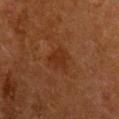This lesion was catalogued during total-body skin photography and was not selected for biopsy.
The tile uses cross-polarized illumination.
The total-body-photography lesion software estimated a border-irregularity rating of about 2.5/10, a within-lesion color-variation index near 1.5/10, and a peripheral color-asymmetry measure near 0.5.
The lesion is located on the chest.
Approximately 3 mm at its widest.
A female patient approximately 50 years of age.
A roughly 15 mm field-of-view crop from a total-body skin photograph.